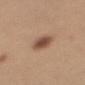Recorded during total-body skin imaging; not selected for excision or biopsy. A female patient, in their 40s. Cropped from a whole-body photographic skin survey; the tile spans about 15 mm. The lesion is on the left upper arm. The lesion-visualizer software estimated an area of roughly 7 mm², a shape eccentricity near 0.7, and two-axis asymmetry of about 0.1. And it measured a lesion color around L≈50 a*≈19 b*≈29 in CIELAB and a lesion–skin lightness drop of about 13. The software also gave border irregularity of about 1.5 on a 0–10 scale, internal color variation of about 4.5 on a 0–10 scale, and peripheral color asymmetry of about 1.5. Measured at roughly 3.5 mm in maximum diameter.